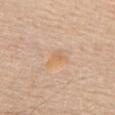A 15 mm close-up tile from a total-body photography series done for melanoma screening. On the back. A male patient in their mid- to late 60s.